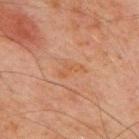Clinical impression:
Imaged during a routine full-body skin examination; the lesion was not biopsied and no histopathology is available.
Context:
A male subject, aged approximately 70. A 15 mm crop from a total-body photograph taken for skin-cancer surveillance. Measured at roughly 3 mm in maximum diameter. The lesion is on the chest.